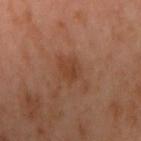Notes:
– workup: no biopsy performed (imaged during a skin exam)
– lighting: cross-polarized illumination
– image-analysis metrics: a footprint of about 5 mm²; a lesion color around L≈42 a*≈23 b*≈32 in CIELAB and roughly 7 lightness units darker than nearby skin
– patient: female, in their mid- to late 50s
– body site: the right upper arm
– size: ≈3 mm
– acquisition: 15 mm crop, total-body photography